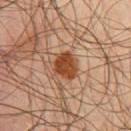Notes:
• patient — male, about 45 years old
• acquisition — total-body-photography crop, ~15 mm field of view
• body site — the front of the torso